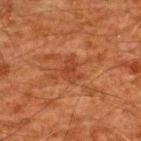| feature | finding |
|---|---|
| notes | imaged on a skin check; not biopsied |
| automated lesion analysis | a shape-asymmetry score of about 0.4 (0 = symmetric); a mean CIELAB color near L≈33 a*≈24 b*≈30 and a normalized border contrast of about 6; a classifier nevus-likeness of about 0/100 |
| site | the upper back |
| subject | male, aged approximately 60 |
| image | ~15 mm tile from a whole-body skin photo |
| lesion diameter | ≈3 mm |
| tile lighting | cross-polarized |